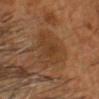Q: Is there a histopathology result?
A: catalogued during a skin exam; not biopsied
Q: What are the patient's age and sex?
A: male, aged 53 to 57
Q: What is the anatomic site?
A: the head or neck
Q: What did automated image analysis measure?
A: two-axis asymmetry of about 0.35; an average lesion color of about L≈35 a*≈18 b*≈30 (CIELAB) and a lesion-to-skin contrast of about 5.5 (normalized; higher = more distinct); a border-irregularity rating of about 5/10 and a within-lesion color-variation index near 3/10; an automated nevus-likeness rating near 0 out of 100 and a lesion-detection confidence of about 100/100
Q: How was this image acquired?
A: 15 mm crop, total-body photography
Q: Illumination type?
A: cross-polarized illumination
Q: Lesion size?
A: ~5.5 mm (longest diameter)